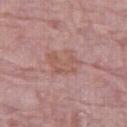| feature | finding |
|---|---|
| workup | no biopsy performed (imaged during a skin exam) |
| site | the right thigh |
| automated metrics | a shape eccentricity near 0.75; border irregularity of about 6 on a 0–10 scale, a color-variation rating of about 2.5/10, and radial color variation of about 1; a detector confidence of about 100 out of 100 that the crop contains a lesion |
| tile lighting | white-light |
| lesion size | about 4 mm |
| image | ~15 mm tile from a whole-body skin photo |
| patient | female, roughly 65 years of age |